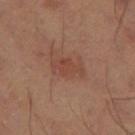Context: Automated tile analysis of the lesion measured a border-irregularity index near 3.5/10. The lesion is located on the left thigh. This is a cross-polarized tile. A close-up tile cropped from a whole-body skin photograph, about 15 mm across.